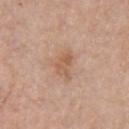Notes:
• follow-up: imaged on a skin check; not biopsied
• TBP lesion metrics: border irregularity of about 4 on a 0–10 scale and a peripheral color-asymmetry measure near 1
• site: the chest
• acquisition: 15 mm crop, total-body photography
• subject: male, aged approximately 60